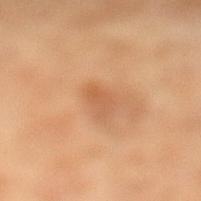The lesion was photographed on a routine skin check and not biopsied; there is no pathology result.
From the left lower leg.
This image is a 15 mm lesion crop taken from a total-body photograph.
A female patient aged approximately 70.